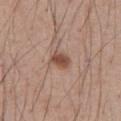Q: Was this lesion biopsied?
A: catalogued during a skin exam; not biopsied
Q: Lesion location?
A: the abdomen
Q: What is the lesion's diameter?
A: ≈2.5 mm
Q: How was this image acquired?
A: ~15 mm crop, total-body skin-cancer survey
Q: What are the patient's age and sex?
A: male, in their 60s
Q: How was the tile lit?
A: white-light